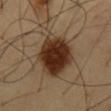Notes:
• biopsy status: imaged on a skin check; not biopsied
• location: the abdomen
• subject: male, aged 58–62
• tile lighting: cross-polarized illumination
• lesion size: ~7 mm (longest diameter)
• image: 15 mm crop, total-body photography